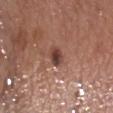No biopsy was performed on this lesion — it was imaged during a full skin examination and was not determined to be concerning.
Measured at roughly 2.5 mm in maximum diameter.
The tile uses white-light illumination.
The lesion is located on the chest.
Cropped from a total-body skin-imaging series; the visible field is about 15 mm.
A male patient, approximately 55 years of age.